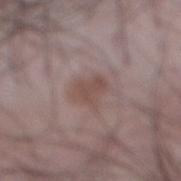lesion_size:
  long_diameter_mm_approx: 3.0
patient:
  sex: male
  age_approx: 45
image:
  source: total-body photography crop
  field_of_view_mm: 15
site: abdomen
lighting: white-light